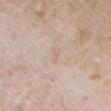Q: How was this image acquired?
A: total-body-photography crop, ~15 mm field of view
Q: Lesion size?
A: ≈2 mm
Q: Who is the patient?
A: male, aged 33 to 37
Q: Illumination type?
A: white-light
Q: Where on the body is the lesion?
A: the head or neck
Q: Automated lesion metrics?
A: a lesion area of about 1.5 mm² and an outline eccentricity of about 0.9 (0 = round, 1 = elongated); an average lesion color of about L≈67 a*≈13 b*≈27 (CIELAB), about 4 CIELAB-L* units darker than the surrounding skin, and a normalized border contrast of about 3.5; a border-irregularity rating of about 4.5/10, a color-variation rating of about 0/10, and a peripheral color-asymmetry measure near 0